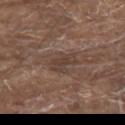biopsy status: imaged on a skin check; not biopsied
anatomic site: the upper back
tile lighting: white-light
image-analysis metrics: an area of roughly 6 mm², an outline eccentricity of about 0.75 (0 = round, 1 = elongated), and two-axis asymmetry of about 0.25; a mean CIELAB color near L≈40 a*≈16 b*≈22 and roughly 7 lightness units darker than nearby skin; a border-irregularity rating of about 3.5/10, a color-variation rating of about 2.5/10, and a peripheral color-asymmetry measure near 1; a classifier nevus-likeness of about 0/100
subject: male, about 80 years old
acquisition: 15 mm crop, total-body photography
lesion size: about 3.5 mm